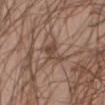Q: What is the imaging modality?
A: 15 mm crop, total-body photography
Q: What are the patient's age and sex?
A: male, in their mid- to late 60s
Q: Where on the body is the lesion?
A: the abdomen